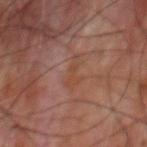Recorded during total-body skin imaging; not selected for excision or biopsy.
Automated tile analysis of the lesion measured a mean CIELAB color near L≈40 a*≈20 b*≈27 and a normalized lesion–skin contrast near 5.5. The software also gave an automated nevus-likeness rating near 0 out of 100 and a detector confidence of about 95 out of 100 that the crop contains a lesion.
The subject is a male in their 60s.
About 3.5 mm across.
Imaged with cross-polarized lighting.
Cropped from a total-body skin-imaging series; the visible field is about 15 mm.
The lesion is located on the upper back.